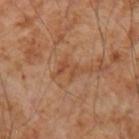This lesion was catalogued during total-body skin photography and was not selected for biopsy. From the right forearm. Cropped from a total-body skin-imaging series; the visible field is about 15 mm. Imaged with cross-polarized lighting. About 3 mm across. A male subject aged 53 to 57.